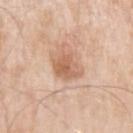Image and clinical context:
The subject is a male roughly 55 years of age. Located on the left upper arm. Automated tile analysis of the lesion measured an area of roughly 8.5 mm² and a shape-asymmetry score of about 0.25 (0 = symmetric). The software also gave a border-irregularity index near 3/10 and internal color variation of about 4.5 on a 0–10 scale. The software also gave a nevus-likeness score of about 10/100 and a detector confidence of about 100 out of 100 that the crop contains a lesion. A roughly 15 mm field-of-view crop from a total-body skin photograph.